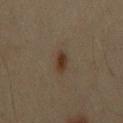<lesion>
  <lesion_size>
    <long_diameter_mm_approx>3.0</long_diameter_mm_approx>
  </lesion_size>
  <patient>
    <sex>male</sex>
    <age_approx>60</age_approx>
  </patient>
  <image>
    <source>total-body photography crop</source>
    <field_of_view_mm>15</field_of_view_mm>
  </image>
  <site>chest</site>
  <lighting>cross-polarized</lighting>
</lesion>